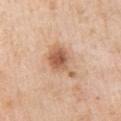workup: total-body-photography surveillance lesion; no biopsy | image-analysis metrics: a mean CIELAB color near L≈60 a*≈21 b*≈34, a lesion–skin lightness drop of about 13, and a lesion-to-skin contrast of about 8 (normalized; higher = more distinct); a classifier nevus-likeness of about 85/100 | anatomic site: the arm | imaging modality: ~15 mm crop, total-body skin-cancer survey | diameter: ≈5 mm | patient: female, in their mid- to late 50s | illumination: white-light.